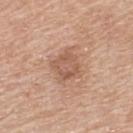{"biopsy_status": "not biopsied; imaged during a skin examination", "lighting": "white-light", "lesion_size": {"long_diameter_mm_approx": 4.0}, "site": "upper back", "patient": {"sex": "male", "age_approx": 60}, "image": {"source": "total-body photography crop", "field_of_view_mm": 15}}